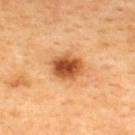Clinical summary: From the upper back. About 4 mm across. A male patient, in their mid- to late 40s. Cropped from a total-body skin-imaging series; the visible field is about 15 mm.